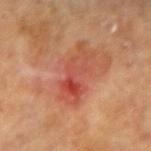notes — no biopsy performed (imaged during a skin exam); image source — ~15 mm crop, total-body skin-cancer survey; site — the left forearm; lighting — cross-polarized illumination; size — ≈6 mm; subject — female, aged around 65.